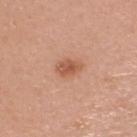Assessment:
Recorded during total-body skin imaging; not selected for excision or biopsy.
Image and clinical context:
A male subject aged 43 to 47. An algorithmic analysis of the crop reported lesion-presence confidence of about 100/100. The lesion is located on the head or neck. Captured under white-light illumination. A lesion tile, about 15 mm wide, cut from a 3D total-body photograph. The recorded lesion diameter is about 3 mm.